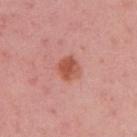Findings:
- workup: no biopsy performed (imaged during a skin exam)
- automated lesion analysis: a border-irregularity rating of about 2/10, internal color variation of about 3.5 on a 0–10 scale, and peripheral color asymmetry of about 1
- tile lighting: white-light illumination
- image: total-body-photography crop, ~15 mm field of view
- subject: female, aged 33–37
- location: the right upper arm